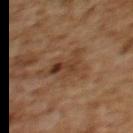The lesion was tiled from a total-body skin photograph and was not biopsied. The subject is a female aged around 60. The lesion is located on the upper back. A close-up tile cropped from a whole-body skin photograph, about 15 mm across. Measured at roughly 4.5 mm in maximum diameter. Imaged with cross-polarized lighting.